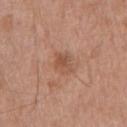Findings:
- workup · no biopsy performed (imaged during a skin exam)
- patient · male, aged 63–67
- location · the chest
- lesion size · about 3 mm
- imaging modality · 15 mm crop, total-body photography